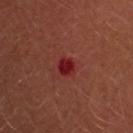{"biopsy_status": "not biopsied; imaged during a skin examination", "site": "head or neck", "patient": {"sex": "male", "age_approx": 45}, "image": {"source": "total-body photography crop", "field_of_view_mm": 15}}